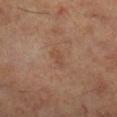Impression:
Imaged during a routine full-body skin examination; the lesion was not biopsied and no histopathology is available.
Acquisition and patient details:
A male subject, roughly 60 years of age. Cropped from a whole-body photographic skin survey; the tile spans about 15 mm. From the right lower leg.